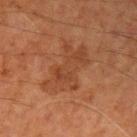Captured during whole-body skin photography for melanoma surveillance; the lesion was not biopsied. The patient is a male in their 70s. Imaged with cross-polarized lighting. Cropped from a whole-body photographic skin survey; the tile spans about 15 mm. The lesion's longest dimension is about 6 mm. Automated tile analysis of the lesion measured a lesion color around L≈36 a*≈21 b*≈29 in CIELAB and roughly 5 lightness units darker than nearby skin. It also reported a border-irregularity index near 6.5/10, a color-variation rating of about 3/10, and radial color variation of about 1. The analysis additionally found a classifier nevus-likeness of about 0/100. On the right upper arm.